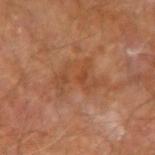illumination: cross-polarized illumination | patient: male, about 65 years old | body site: the left forearm | image source: 15 mm crop, total-body photography | automated lesion analysis: a mean CIELAB color near L≈45 a*≈23 b*≈34 and a normalized lesion–skin contrast near 5.5.